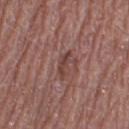biopsy status: catalogued during a skin exam; not biopsied | patient: male, in their 70s | automated metrics: a lesion color around L≈41 a*≈21 b*≈22 in CIELAB and about 9 CIELAB-L* units darker than the surrounding skin; border irregularity of about 5 on a 0–10 scale, a within-lesion color-variation index near 0.5/10, and peripheral color asymmetry of about 0; a nevus-likeness score of about 0/100 and a lesion-detection confidence of about 80/100 | size: ≈3 mm | illumination: white-light illumination | imaging modality: 15 mm crop, total-body photography | anatomic site: the right thigh.